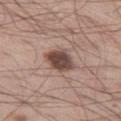workup: total-body-photography surveillance lesion; no biopsy | illumination: white-light | subject: male, roughly 55 years of age | image-analysis metrics: a footprint of about 8 mm² and a symmetry-axis asymmetry near 0.15; a lesion color around L≈46 a*≈18 b*≈22 in CIELAB, a lesion–skin lightness drop of about 16, and a lesion-to-skin contrast of about 11.5 (normalized; higher = more distinct); a border-irregularity index near 1.5/10, a within-lesion color-variation index near 3.5/10, and a peripheral color-asymmetry measure near 1 | image source: total-body-photography crop, ~15 mm field of view | diameter: about 3.5 mm | body site: the left thigh.